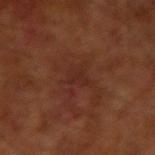Imaged during a routine full-body skin examination; the lesion was not biopsied and no histopathology is available. An algorithmic analysis of the crop reported a lesion area of about 3.5 mm², an outline eccentricity of about 0.65 (0 = round, 1 = elongated), and a symmetry-axis asymmetry near 0.35. The software also gave an average lesion color of about L≈26 a*≈22 b*≈24 (CIELAB), roughly 4 lightness units darker than nearby skin, and a lesion-to-skin contrast of about 4.5 (normalized; higher = more distinct). And it measured border irregularity of about 3 on a 0–10 scale, internal color variation of about 1.5 on a 0–10 scale, and peripheral color asymmetry of about 0.5. The software also gave a nevus-likeness score of about 0/100 and a lesion-detection confidence of about 100/100. Cropped from a whole-body photographic skin survey; the tile spans about 15 mm. From the right upper arm. The tile uses cross-polarized illumination. A male patient in their mid-60s.